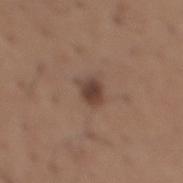Assessment:
The lesion was tiled from a total-body skin photograph and was not biopsied.
Image and clinical context:
A 15 mm close-up extracted from a 3D total-body photography capture. The lesion is located on the mid back. The tile uses white-light illumination. Approximately 2.5 mm at its widest. A male subject in their mid-60s.